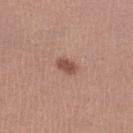This lesion was catalogued during total-body skin photography and was not selected for biopsy. A 15 mm close-up tile from a total-body photography series done for melanoma screening. The total-body-photography lesion software estimated a classifier nevus-likeness of about 90/100. A female subject, aged 33 to 37. On the left lower leg. Imaged with white-light lighting. The lesion's longest dimension is about 2.5 mm.